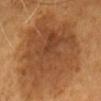Clinical impression:
This lesion was catalogued during total-body skin photography and was not selected for biopsy.
Clinical summary:
Cropped from a whole-body photographic skin survey; the tile spans about 15 mm. The lesion is located on the head or neck. A female subject aged around 60. This is a cross-polarized tile.